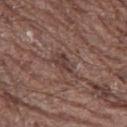workup=catalogued during a skin exam; not biopsied | tile lighting=white-light illumination | diameter=~4 mm (longest diameter) | image source=total-body-photography crop, ~15 mm field of view | site=the left thigh | patient=male, in their 70s.